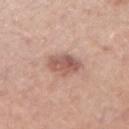Part of a total-body skin-imaging series; this lesion was reviewed on a skin check and was not flagged for biopsy. A male subject approximately 40 years of age. The lesion's longest dimension is about 4 mm. This image is a 15 mm lesion crop taken from a total-body photograph. The total-body-photography lesion software estimated a footprint of about 9 mm², an outline eccentricity of about 0.7 (0 = round, 1 = elongated), and two-axis asymmetry of about 0.15. It also reported internal color variation of about 4.5 on a 0–10 scale and radial color variation of about 1.5. From the right upper arm.